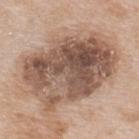Case summary:
– follow-up · catalogued during a skin exam; not biopsied
– subject · male, aged around 65
– site · the upper back
– automated metrics · border irregularity of about 2.5 on a 0–10 scale, a within-lesion color-variation index near 9/10, and a peripheral color-asymmetry measure near 3.5; a classifier nevus-likeness of about 10/100 and a detector confidence of about 100 out of 100 that the crop contains a lesion
– image source · 15 mm crop, total-body photography